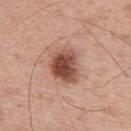Q: Is there a histopathology result?
A: total-body-photography surveillance lesion; no biopsy
Q: How large is the lesion?
A: ≈4 mm
Q: What are the patient's age and sex?
A: male, aged 63–67
Q: Lesion location?
A: the upper back
Q: What kind of image is this?
A: ~15 mm crop, total-body skin-cancer survey